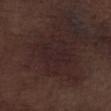{"image": {"source": "total-body photography crop", "field_of_view_mm": 15}, "patient": {"sex": "male", "age_approx": 70}, "lighting": "white-light", "site": "left lower leg"}